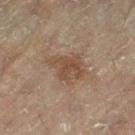workup: no biopsy performed (imaged during a skin exam)
subject: female, aged around 80
diameter: about 4 mm
image-analysis metrics: a shape eccentricity near 0.6 and a shape-asymmetry score of about 0.3 (0 = symmetric); lesion-presence confidence of about 100/100
imaging modality: ~15 mm crop, total-body skin-cancer survey
tile lighting: cross-polarized illumination
site: the right leg